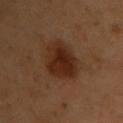Impression: The lesion was photographed on a routine skin check and not biopsied; there is no pathology result. Acquisition and patient details: A female subject, in their 60s. Cropped from a whole-body photographic skin survey; the tile spans about 15 mm. From the chest.